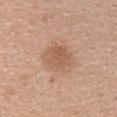Part of a total-body skin-imaging series; this lesion was reviewed on a skin check and was not flagged for biopsy. The subject is a female aged 23 to 27. Cropped from a total-body skin-imaging series; the visible field is about 15 mm. From the upper back. Imaged with white-light lighting.